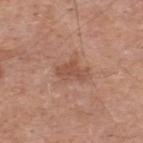{"biopsy_status": "not biopsied; imaged during a skin examination", "site": "back", "lighting": "white-light", "image": {"source": "total-body photography crop", "field_of_view_mm": 15}, "patient": {"sex": "male", "age_approx": 55}}